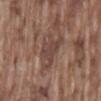biopsy status = total-body-photography surveillance lesion; no biopsy | anatomic site = the lower back | lesion diameter = ~4.5 mm (longest diameter) | subject = male, aged 73 to 77 | acquisition = 15 mm crop, total-body photography.